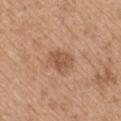- biopsy status: no biopsy performed (imaged during a skin exam)